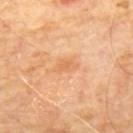  biopsy_status: not biopsied; imaged during a skin examination
  patient:
    sex: male
    age_approx: 70
  image:
    source: total-body photography crop
    field_of_view_mm: 15
  lesion_size:
    long_diameter_mm_approx: 2.5
  site: back
  lighting: cross-polarized
  automated_metrics:
    border_irregularity_0_10: 3.5
    peripheral_color_asymmetry: 1.0
    nevus_likeness_0_100: 0
    lesion_detection_confidence_0_100: 100